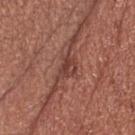Captured during whole-body skin photography for melanoma surveillance; the lesion was not biopsied.
Located on the head or neck.
A region of skin cropped from a whole-body photographic capture, roughly 15 mm wide.
A male subject, aged 53 to 57.